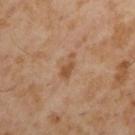follow-up = total-body-photography surveillance lesion; no biopsy | patient = male, roughly 55 years of age | TBP lesion metrics = border irregularity of about 4.5 on a 0–10 scale and a color-variation rating of about 1/10 | acquisition = ~15 mm crop, total-body skin-cancer survey | location = the arm | lighting = cross-polarized illumination.